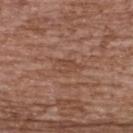Part of a total-body skin-imaging series; this lesion was reviewed on a skin check and was not flagged for biopsy. Located on the upper back. A female patient aged approximately 65. The lesion-visualizer software estimated an area of roughly 4 mm², an outline eccentricity of about 0.8 (0 = round, 1 = elongated), and two-axis asymmetry of about 0.25. It also reported a border-irregularity index near 2.5/10, a within-lesion color-variation index near 2/10, and peripheral color asymmetry of about 0.5. This image is a 15 mm lesion crop taken from a total-body photograph.